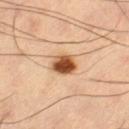The patient is a male aged approximately 60.
Captured under cross-polarized illumination.
Longest diameter approximately 3 mm.
From the left thigh.
Cropped from a whole-body photographic skin survey; the tile spans about 15 mm.